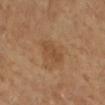This lesion was catalogued during total-body skin photography and was not selected for biopsy.